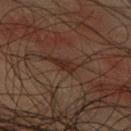No biopsy was performed on this lesion — it was imaged during a full skin examination and was not determined to be concerning. The total-body-photography lesion software estimated a mean CIELAB color near L≈20 a*≈15 b*≈18, a lesion–skin lightness drop of about 6, and a normalized lesion–skin contrast near 7. A male subject aged 48 to 52. Imaged with cross-polarized lighting. Cropped from a total-body skin-imaging series; the visible field is about 15 mm. From the upper back. The recorded lesion diameter is about 2.5 mm.